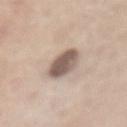The recorded lesion diameter is about 4.5 mm.
Located on the chest.
The tile uses white-light illumination.
Automated image analysis of the tile measured a lesion–skin lightness drop of about 16 and a lesion-to-skin contrast of about 10.5 (normalized; higher = more distinct). The analysis additionally found a border-irregularity rating of about 1.5/10, internal color variation of about 3.5 on a 0–10 scale, and radial color variation of about 1. It also reported an automated nevus-likeness rating near 85 out of 100 and a lesion-detection confidence of about 95/100.
A male patient, approximately 60 years of age.
A close-up tile cropped from a whole-body skin photograph, about 15 mm across.